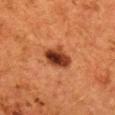Assessment:
The lesion was photographed on a routine skin check and not biopsied; there is no pathology result.
Image and clinical context:
The patient is a female about 50 years old. Automated image analysis of the tile measured a lesion color around L≈33 a*≈25 b*≈31 in CIELAB, a lesion–skin lightness drop of about 15, and a lesion-to-skin contrast of about 12.5 (normalized; higher = more distinct). The software also gave a nevus-likeness score of about 95/100 and a detector confidence of about 100 out of 100 that the crop contains a lesion. Cropped from a whole-body photographic skin survey; the tile spans about 15 mm. Approximately 4 mm at its widest. This is a cross-polarized tile. On the mid back.